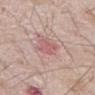This lesion was catalogued during total-body skin photography and was not selected for biopsy.
An algorithmic analysis of the crop reported a lesion color around L≈58 a*≈25 b*≈21 in CIELAB and a normalized lesion–skin contrast near 5.5. The analysis additionally found a within-lesion color-variation index near 2/10 and radial color variation of about 0.5.
Located on the abdomen.
This image is a 15 mm lesion crop taken from a total-body photograph.
A male subject aged around 65.
The recorded lesion diameter is about 3 mm.